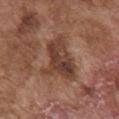Assessment:
Captured during whole-body skin photography for melanoma surveillance; the lesion was not biopsied.
Acquisition and patient details:
Imaged with white-light lighting. Located on the front of the torso. A male subject in their mid- to late 70s. Automated tile analysis of the lesion measured a lesion color around L≈40 a*≈20 b*≈27 in CIELAB and a lesion-to-skin contrast of about 8.5 (normalized; higher = more distinct). The software also gave a border-irregularity index near 4.5/10, internal color variation of about 5.5 on a 0–10 scale, and peripheral color asymmetry of about 2. Cropped from a whole-body photographic skin survey; the tile spans about 15 mm.